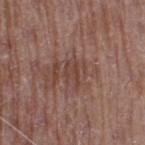biopsy status: imaged on a skin check; not biopsied | site: the right thigh | patient: male, in their 70s | lesion size: about 3 mm | illumination: white-light illumination | imaging modality: total-body-photography crop, ~15 mm field of view.